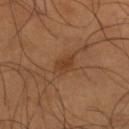Captured during whole-body skin photography for melanoma surveillance; the lesion was not biopsied. The lesion is on the leg. This image is a 15 mm lesion crop taken from a total-body photograph. A male patient aged 53–57.